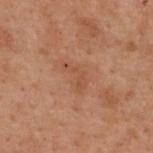follow-up: imaged on a skin check; not biopsied | location: the upper back | acquisition: 15 mm crop, total-body photography | patient: female, aged approximately 55 | lighting: cross-polarized | automated metrics: an eccentricity of roughly 0.9 and two-axis asymmetry of about 0.55; a mean CIELAB color near L≈41 a*≈20 b*≈29, roughly 5 lightness units darker than nearby skin, and a lesion-to-skin contrast of about 4.5 (normalized; higher = more distinct); a color-variation rating of about 1/10 and a peripheral color-asymmetry measure near 0.5 | diameter: about 3.5 mm.